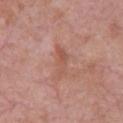A close-up tile cropped from a whole-body skin photograph, about 15 mm across.
The lesion is located on the chest.
The recorded lesion diameter is about 4 mm.
The tile uses white-light illumination.
The subject is a male aged approximately 55.